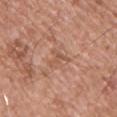No biopsy was performed on this lesion — it was imaged during a full skin examination and was not determined to be concerning.